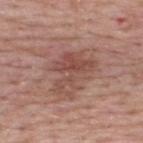Assessment: Captured during whole-body skin photography for melanoma surveillance; the lesion was not biopsied. Image and clinical context: A male subject, in their mid-50s. About 5.5 mm across. Imaged with white-light lighting. On the upper back. A close-up tile cropped from a whole-body skin photograph, about 15 mm across.